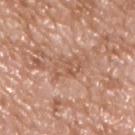<case>
<biopsy_status>not biopsied; imaged during a skin examination</biopsy_status>
<lighting>white-light</lighting>
<site>front of the torso</site>
<patient>
  <sex>male</sex>
  <age_approx>50</age_approx>
</patient>
<image>
  <source>total-body photography crop</source>
  <field_of_view_mm>15</field_of_view_mm>
</image>
<lesion_size>
  <long_diameter_mm_approx>3.5</long_diameter_mm_approx>
</lesion_size>
</case>